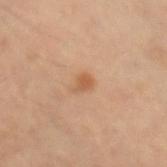Findings:
- workup: catalogued during a skin exam; not biopsied
- lighting: cross-polarized
- TBP lesion metrics: an area of roughly 3 mm², a shape eccentricity near 0.6, and a shape-asymmetry score of about 0.35 (0 = symmetric); an average lesion color of about L≈55 a*≈21 b*≈35 (CIELAB) and a lesion–skin lightness drop of about 8; an automated nevus-likeness rating near 75 out of 100 and a detector confidence of about 100 out of 100 that the crop contains a lesion
- size: about 2.5 mm
- image source: 15 mm crop, total-body photography
- patient: male, about 55 years old
- location: the arm